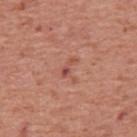Assessment: No biopsy was performed on this lesion — it was imaged during a full skin examination and was not determined to be concerning. Context: This is a white-light tile. Cropped from a whole-body photographic skin survey; the tile spans about 15 mm. The lesion is located on the back. The subject is a male about 70 years old.